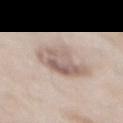Part of a total-body skin-imaging series; this lesion was reviewed on a skin check and was not flagged for biopsy. A roughly 15 mm field-of-view crop from a total-body skin photograph. On the front of the torso. The recorded lesion diameter is about 4.5 mm. The patient is a male aged 53 to 57. Imaged with white-light lighting.